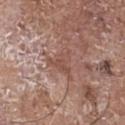This lesion was catalogued during total-body skin photography and was not selected for biopsy. Cropped from a total-body skin-imaging series; the visible field is about 15 mm. The recorded lesion diameter is about 3.5 mm. The tile uses white-light illumination. Located on the chest. A male patient aged 68–72.